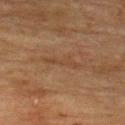• follow-up: catalogued during a skin exam; not biopsied
• site: the upper back
• image: ~15 mm tile from a whole-body skin photo
• size: about 4 mm
• subject: male, in their mid- to late 70s